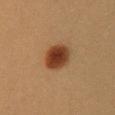No biopsy was performed on this lesion — it was imaged during a full skin examination and was not determined to be concerning. The lesion-visualizer software estimated an area of roughly 10 mm², a shape eccentricity near 0.6, and a symmetry-axis asymmetry near 0.05. And it measured a lesion color around L≈35 a*≈20 b*≈30 in CIELAB, a lesion–skin lightness drop of about 13, and a lesion-to-skin contrast of about 11.5 (normalized; higher = more distinct). The software also gave lesion-presence confidence of about 100/100. Longest diameter approximately 4 mm. On the chest. A female subject, aged approximately 40. A 15 mm close-up tile from a total-body photography series done for melanoma screening.